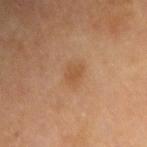Q: Was this lesion biopsied?
A: catalogued during a skin exam; not biopsied
Q: Who is the patient?
A: female, aged approximately 55
Q: Lesion size?
A: ~3 mm (longest diameter)
Q: What is the anatomic site?
A: the right upper arm
Q: How was this image acquired?
A: ~15 mm crop, total-body skin-cancer survey